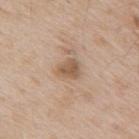Findings:
• workup · no biopsy performed (imaged during a skin exam)
• subject · male, roughly 65 years of age
• imaging modality · ~15 mm crop, total-body skin-cancer survey
• anatomic site · the upper back
• automated metrics · a footprint of about 5 mm² and a shape eccentricity near 0.45; an automated nevus-likeness rating near 10 out of 100 and lesion-presence confidence of about 100/100
• tile lighting · white-light illumination